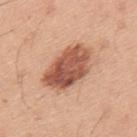The lesion was tiled from a total-body skin photograph and was not biopsied. The lesion is located on the back. A male patient aged around 45. Automated tile analysis of the lesion measured a lesion–skin lightness drop of about 16 and a normalized border contrast of about 10.5. The analysis additionally found a nevus-likeness score of about 60/100 and a detector confidence of about 100 out of 100 that the crop contains a lesion. The lesion's longest dimension is about 6.5 mm. The tile uses white-light illumination. A lesion tile, about 15 mm wide, cut from a 3D total-body photograph.